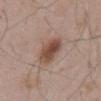Measured at roughly 4.5 mm in maximum diameter. An algorithmic analysis of the crop reported an area of roughly 10 mm², an eccentricity of roughly 0.7, and a shape-asymmetry score of about 0.15 (0 = symmetric). It also reported internal color variation of about 5.5 on a 0–10 scale and radial color variation of about 2. The patient is a male in their mid-50s. A lesion tile, about 15 mm wide, cut from a 3D total-body photograph.